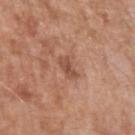Impression:
Recorded during total-body skin imaging; not selected for excision or biopsy.
Acquisition and patient details:
A male patient, in their mid- to late 50s. Automated image analysis of the tile measured an area of roughly 3.5 mm², an outline eccentricity of about 0.8 (0 = round, 1 = elongated), and two-axis asymmetry of about 0.25. It also reported a mean CIELAB color near L≈50 a*≈23 b*≈29, about 10 CIELAB-L* units darker than the surrounding skin, and a normalized lesion–skin contrast near 7. It also reported border irregularity of about 2.5 on a 0–10 scale, a color-variation rating of about 1.5/10, and peripheral color asymmetry of about 0.5. On the left upper arm. A 15 mm close-up tile from a total-body photography series done for melanoma screening. Captured under white-light illumination.